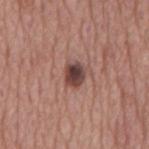Notes:
– notes · no biopsy performed (imaged during a skin exam)
– anatomic site · the mid back
– size · ≈3 mm
– patient · male, in their mid- to late 70s
– automated lesion analysis · a footprint of about 5.5 mm², an eccentricity of roughly 0.55, and a shape-asymmetry score of about 0.25 (0 = symmetric); border irregularity of about 2 on a 0–10 scale, a color-variation rating of about 5/10, and peripheral color asymmetry of about 1.5
– image source · ~15 mm crop, total-body skin-cancer survey
– tile lighting · white-light